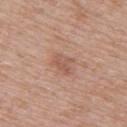Notes:
– notes · no biopsy performed (imaged during a skin exam)
– image source · ~15 mm tile from a whole-body skin photo
– site · the right upper arm
– tile lighting · white-light illumination
– subject · female, in their 30s
– lesion size · ~2.5 mm (longest diameter)